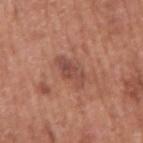Impression:
This lesion was catalogued during total-body skin photography and was not selected for biopsy.
Acquisition and patient details:
Located on the right upper arm. Automated image analysis of the tile measured an area of roughly 6 mm², a shape eccentricity near 0.9, and two-axis asymmetry of about 0.35. It also reported a lesion–skin lightness drop of about 9 and a normalized border contrast of about 6.5. The analysis additionally found a border-irregularity index near 4/10, a within-lesion color-variation index near 4/10, and peripheral color asymmetry of about 1.5. And it measured an automated nevus-likeness rating near 15 out of 100. The recorded lesion diameter is about 4 mm. Captured under white-light illumination. This image is a 15 mm lesion crop taken from a total-body photograph. A male subject, in their mid-70s.